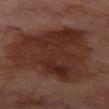<tbp_lesion>
<image>
  <source>total-body photography crop</source>
  <field_of_view_mm>15</field_of_view_mm>
</image>
<site>left thigh</site>
<patient>
  <sex>male</sex>
  <age_approx>70</age_approx>
</patient>
<lighting>cross-polarized</lighting>
<automated_metrics>
  <border_irregularity_0_10>4.0</border_irregularity_0_10>
</automated_metrics>
</tbp_lesion>